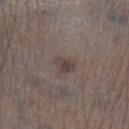anatomic site: the leg | subject: male, roughly 70 years of age | diameter: ≈2.5 mm | image: ~15 mm tile from a whole-body skin photo | TBP lesion metrics: a footprint of about 3.5 mm², a shape eccentricity near 0.75, and two-axis asymmetry of about 0.25; an average lesion color of about L≈40 a*≈12 b*≈17 (CIELAB) and roughly 8 lightness units darker than nearby skin; peripheral color asymmetry of about 0.5; a classifier nevus-likeness of about 15/100 and a lesion-detection confidence of about 100/100.